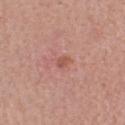Q: Was a biopsy performed?
A: total-body-photography surveillance lesion; no biopsy
Q: Who is the patient?
A: female, aged 43 to 47
Q: What is the anatomic site?
A: the head or neck
Q: How was this image acquired?
A: ~15 mm crop, total-body skin-cancer survey